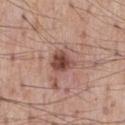Assessment: Captured during whole-body skin photography for melanoma surveillance; the lesion was not biopsied. Background: A lesion tile, about 15 mm wide, cut from a 3D total-body photograph. Automated image analysis of the tile measured a lesion area of about 6.5 mm², an outline eccentricity of about 0.45 (0 = round, 1 = elongated), and a symmetry-axis asymmetry near 0.25. And it measured roughly 13 lightness units darker than nearby skin and a normalized lesion–skin contrast near 9.5. The lesion's longest dimension is about 3 mm. A male subject aged 53 to 57. Imaged with white-light lighting. The lesion is located on the chest.